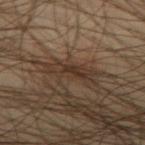biopsy status = catalogued during a skin exam; not biopsied
imaging modality = total-body-photography crop, ~15 mm field of view
lesion size = about 6 mm
automated lesion analysis = a footprint of about 8.5 mm², an eccentricity of roughly 0.95, and two-axis asymmetry of about 0.35; a mean CIELAB color near L≈32 a*≈14 b*≈24, about 7 CIELAB-L* units darker than the surrounding skin, and a normalized border contrast of about 7; border irregularity of about 6 on a 0–10 scale, a within-lesion color-variation index near 3.5/10, and radial color variation of about 1; a nevus-likeness score of about 0/100 and lesion-presence confidence of about 15/100
body site = the leg
subject = male, in their mid- to late 40s
illumination = cross-polarized illumination